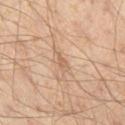diameter: ≈2.5 mm | image-analysis metrics: a footprint of about 2.5 mm² and an outline eccentricity of about 0.9 (0 = round, 1 = elongated); a lesion color around L≈60 a*≈18 b*≈31 in CIELAB and a normalized border contrast of about 5.5; a border-irregularity index near 3/10, internal color variation of about 0.5 on a 0–10 scale, and a peripheral color-asymmetry measure near 0 | imaging modality: ~15 mm tile from a whole-body skin photo | anatomic site: the left thigh | tile lighting: cross-polarized | patient: male, roughly 45 years of age.